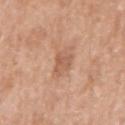Recorded during total-body skin imaging; not selected for excision or biopsy. Captured under white-light illumination. The subject is a male aged around 65. About 3 mm across. From the right upper arm. Automated tile analysis of the lesion measured a shape eccentricity near 0.4 and two-axis asymmetry of about 0.25. A 15 mm close-up extracted from a 3D total-body photography capture.